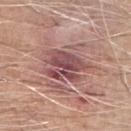Impression:
No biopsy was performed on this lesion — it was imaged during a full skin examination and was not determined to be concerning.
Background:
The recorded lesion diameter is about 5.5 mm. The patient is a male aged 63–67. This image is a 15 mm lesion crop taken from a total-body photograph. Located on the right forearm. The tile uses white-light illumination.